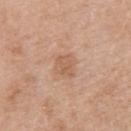Impression:
The lesion was tiled from a total-body skin photograph and was not biopsied.
Clinical summary:
A close-up tile cropped from a whole-body skin photograph, about 15 mm across. The lesion-visualizer software estimated a border-irregularity rating of about 3/10, internal color variation of about 2 on a 0–10 scale, and a peripheral color-asymmetry measure near 0.5. It also reported a nevus-likeness score of about 0/100 and a detector confidence of about 100 out of 100 that the crop contains a lesion. Located on the left upper arm. The lesion's longest dimension is about 2.5 mm. A male subject aged 63–67. The tile uses white-light illumination.